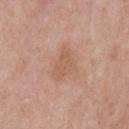Imaged during a routine full-body skin examination; the lesion was not biopsied and no histopathology is available. The tile uses white-light illumination. The lesion is on the chest. An algorithmic analysis of the crop reported a lesion area of about 6.5 mm², an eccentricity of roughly 0.85, and a shape-asymmetry score of about 0.35 (0 = symmetric). And it measured a border-irregularity rating of about 3.5/10, a within-lesion color-variation index near 2/10, and radial color variation of about 1. Approximately 4 mm at its widest. The subject is a male aged around 55. This image is a 15 mm lesion crop taken from a total-body photograph.